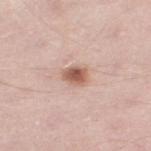Q: Is there a histopathology result?
A: no biopsy performed (imaged during a skin exam)
Q: How was the tile lit?
A: white-light
Q: Where on the body is the lesion?
A: the left lower leg
Q: How was this image acquired?
A: 15 mm crop, total-body photography
Q: Patient demographics?
A: male, aged 48–52
Q: Automated lesion metrics?
A: a lesion area of about 4.5 mm² and two-axis asymmetry of about 0.2; a lesion color around L≈58 a*≈22 b*≈28 in CIELAB, a lesion–skin lightness drop of about 15, and a lesion-to-skin contrast of about 9.5 (normalized; higher = more distinct); a within-lesion color-variation index near 4/10
Q: Lesion size?
A: ≈2.5 mm